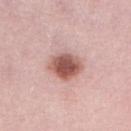Recorded during total-body skin imaging; not selected for excision or biopsy. The patient is a female aged 38–42. Imaged with white-light lighting. This image is a 15 mm lesion crop taken from a total-body photograph. On the lower back. Longest diameter approximately 4 mm.